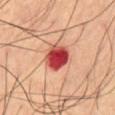Assessment: The lesion was photographed on a routine skin check and not biopsied; there is no pathology result. Clinical summary: From the abdomen. A male subject aged around 70. A roughly 15 mm field-of-view crop from a total-body skin photograph. The tile uses cross-polarized illumination. Automated tile analysis of the lesion measured an average lesion color of about L≈47 a*≈39 b*≈30 (CIELAB) and a normalized border contrast of about 13.5. About 3.5 mm across.